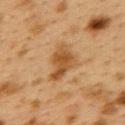Findings:
- workup · no biopsy performed (imaged during a skin exam)
- illumination · cross-polarized
- diameter · ~5 mm (longest diameter)
- image · 15 mm crop, total-body photography
- anatomic site · the back
- patient · female, aged approximately 40